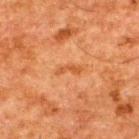Q: Was this lesion biopsied?
A: catalogued during a skin exam; not biopsied
Q: What are the patient's age and sex?
A: male, aged 58 to 62
Q: What lighting was used for the tile?
A: cross-polarized illumination
Q: Lesion location?
A: the upper back
Q: Lesion size?
A: about 3 mm
Q: What kind of image is this?
A: 15 mm crop, total-body photography
Q: What did automated image analysis measure?
A: a symmetry-axis asymmetry near 0.5; border irregularity of about 5.5 on a 0–10 scale; an automated nevus-likeness rating near 0 out of 100 and lesion-presence confidence of about 100/100